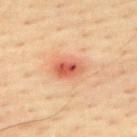Part of a total-body skin-imaging series; this lesion was reviewed on a skin check and was not flagged for biopsy.
A roughly 15 mm field-of-view crop from a total-body skin photograph.
The lesion's longest dimension is about 4 mm.
The patient is a male aged around 65.
This is a cross-polarized tile.
The lesion is located on the upper back.